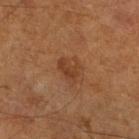{"biopsy_status": "not biopsied; imaged during a skin examination", "image": {"source": "total-body photography crop", "field_of_view_mm": 15}, "site": "left leg", "lighting": "cross-polarized", "patient": {"sex": "male", "age_approx": 65}, "lesion_size": {"long_diameter_mm_approx": 3.0}}